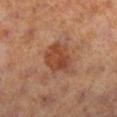Imaged with cross-polarized lighting.
This image is a 15 mm lesion crop taken from a total-body photograph.
About 4.5 mm across.
The lesion-visualizer software estimated a classifier nevus-likeness of about 50/100 and lesion-presence confidence of about 100/100.
The lesion is on the right lower leg.
The patient is a female aged around 40.